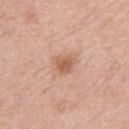{"image": {"source": "total-body photography crop", "field_of_view_mm": 15}, "patient": {"sex": "male", "age_approx": 80}, "lighting": "white-light", "site": "right upper arm", "lesion_size": {"long_diameter_mm_approx": 2.5}}